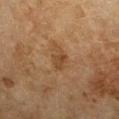biopsy_status: not biopsied; imaged during a skin examination
site: left forearm
image:
  source: total-body photography crop
  field_of_view_mm: 15
lesion_size:
  long_diameter_mm_approx: 3.0
lighting: cross-polarized
patient:
  sex: female
  age_approx: 60